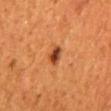| key | value |
|---|---|
| lighting | cross-polarized |
| site | the mid back |
| acquisition | ~15 mm crop, total-body skin-cancer survey |
| subject | male, aged 43–47 |